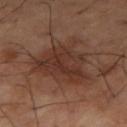Assessment: The lesion was tiled from a total-body skin photograph and was not biopsied.